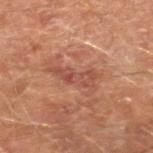• biopsy status — no biopsy performed (imaged during a skin exam)
• image source — total-body-photography crop, ~15 mm field of view
• location — the right lower leg
• patient — male, aged approximately 65
• lighting — cross-polarized
• size — ~5 mm (longest diameter)